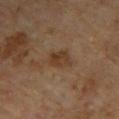No biopsy was performed on this lesion — it was imaged during a full skin examination and was not determined to be concerning. The lesion is on the right upper arm. A male patient, in their mid-60s. Imaged with cross-polarized lighting. An algorithmic analysis of the crop reported a mean CIELAB color near L≈35 a*≈17 b*≈29 and a lesion–skin lightness drop of about 8. It also reported border irregularity of about 2.5 on a 0–10 scale, a within-lesion color-variation index near 3.5/10, and peripheral color asymmetry of about 1. The analysis additionally found an automated nevus-likeness rating near 20 out of 100 and lesion-presence confidence of about 100/100. Cropped from a total-body skin-imaging series; the visible field is about 15 mm. The recorded lesion diameter is about 3 mm.